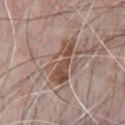The lesion was photographed on a routine skin check and not biopsied; there is no pathology result. A roughly 15 mm field-of-view crop from a total-body skin photograph. The lesion is located on the chest. The recorded lesion diameter is about 6.5 mm. Automated tile analysis of the lesion measured roughly 10 lightness units darker than nearby skin and a lesion-to-skin contrast of about 8 (normalized; higher = more distinct). It also reported a border-irregularity index near 7.5/10, a within-lesion color-variation index near 4.5/10, and peripheral color asymmetry of about 1.5. It also reported a nevus-likeness score of about 0/100 and a lesion-detection confidence of about 95/100. A male patient roughly 70 years of age.